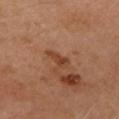  biopsy_status: not biopsied; imaged during a skin examination
  lesion_size:
    long_diameter_mm_approx: 2.5
  lighting: cross-polarized
  site: head or neck
  patient:
    sex: female
    age_approx: 60
  automated_metrics:
    area_mm2_approx: 3.5
    eccentricity: 0.8
    shape_asymmetry: 0.25
    cielab_L: 36
    cielab_a: 21
    cielab_b: 29
    vs_skin_darker_L: 7.0
    border_irregularity_0_10: 2.5
    color_variation_0_10: 2.5
    peripheral_color_asymmetry: 1.0
  image:
    source: total-body photography crop
    field_of_view_mm: 15